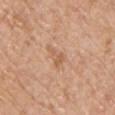No biopsy was performed on this lesion — it was imaged during a full skin examination and was not determined to be concerning. A lesion tile, about 15 mm wide, cut from a 3D total-body photograph. About 3 mm across. On the chest. The total-body-photography lesion software estimated an area of roughly 3 mm² and a shape-asymmetry score of about 0.6 (0 = symmetric). And it measured an average lesion color of about L≈59 a*≈21 b*≈34 (CIELAB), a lesion–skin lightness drop of about 8, and a normalized lesion–skin contrast near 5.5. And it measured an automated nevus-likeness rating near 0 out of 100 and a detector confidence of about 100 out of 100 that the crop contains a lesion. This is a white-light tile. A male subject, aged 58–62.